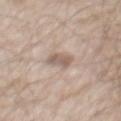notes: catalogued during a skin exam; not biopsied | acquisition: ~15 mm crop, total-body skin-cancer survey | lighting: white-light illumination | size: ≈2.5 mm | automated lesion analysis: a lesion area of about 4.5 mm² and an outline eccentricity of about 0.4 (0 = round, 1 = elongated); roughly 10 lightness units darker than nearby skin and a lesion-to-skin contrast of about 7 (normalized; higher = more distinct); a border-irregularity rating of about 2/10 and a peripheral color-asymmetry measure near 0.5; a lesion-detection confidence of about 70/100 | patient: male, aged around 65 | location: the back.